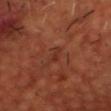<case>
  <biopsy_status>not biopsied; imaged during a skin examination</biopsy_status>
  <image>
    <source>total-body photography crop</source>
    <field_of_view_mm>15</field_of_view_mm>
  </image>
  <lighting>cross-polarized</lighting>
  <lesion_size>
    <long_diameter_mm_approx>3.0</long_diameter_mm_approx>
  </lesion_size>
  <site>head or neck</site>
  <patient>
    <sex>male</sex>
    <age_approx>50</age_approx>
  </patient>
</case>